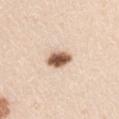This lesion was catalogued during total-body skin photography and was not selected for biopsy. A 15 mm close-up tile from a total-body photography series done for melanoma screening. A male subject aged 43–47. Approximately 3.5 mm at its widest. Located on the left upper arm. Captured under white-light illumination. The total-body-photography lesion software estimated a shape eccentricity near 0.7 and a symmetry-axis asymmetry near 0.2. The analysis additionally found a lesion color around L≈60 a*≈18 b*≈30 in CIELAB, roughly 22 lightness units darker than nearby skin, and a normalized lesion–skin contrast near 12.5. And it measured border irregularity of about 2 on a 0–10 scale, internal color variation of about 5 on a 0–10 scale, and peripheral color asymmetry of about 1.5. And it measured an automated nevus-likeness rating near 100 out of 100.